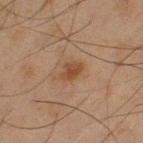{"biopsy_status": "not biopsied; imaged during a skin examination", "patient": {"sex": "male", "age_approx": 45}, "image": {"source": "total-body photography crop", "field_of_view_mm": 15}, "site": "left thigh", "lighting": "cross-polarized", "automated_metrics": {"area_mm2_approx": 4.0, "eccentricity": 0.75, "shape_asymmetry": 0.3, "cielab_L": 37, "cielab_a": 17, "cielab_b": 28, "vs_skin_darker_L": 7.0, "vs_skin_contrast_norm": 7.5, "nevus_likeness_0_100": 80, "lesion_detection_confidence_0_100": 100}, "lesion_size": {"long_diameter_mm_approx": 2.5}}